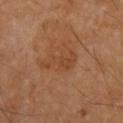Q: Is there a histopathology result?
A: total-body-photography surveillance lesion; no biopsy
Q: How was this image acquired?
A: 15 mm crop, total-body photography
Q: Who is the patient?
A: male, in their mid- to late 50s
Q: What is the anatomic site?
A: the left upper arm
Q: What is the lesion's diameter?
A: ~5 mm (longest diameter)
Q: Illumination type?
A: cross-polarized